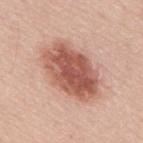On the mid back.
Captured under white-light illumination.
The recorded lesion diameter is about 8 mm.
The subject is a male aged around 60.
The total-body-photography lesion software estimated a lesion color around L≈56 a*≈25 b*≈28 in CIELAB, roughly 15 lightness units darker than nearby skin, and a normalized border contrast of about 9.5.
A close-up tile cropped from a whole-body skin photograph, about 15 mm across.